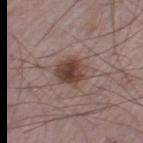Case summary:
– biopsy status · catalogued during a skin exam; not biopsied
– patient · male, aged around 75
– anatomic site · the left thigh
– lighting · white-light
– image · ~15 mm crop, total-body skin-cancer survey
– lesion diameter · ≈3.5 mm